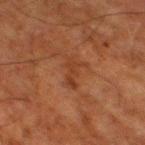Case summary:
• notes: total-body-photography surveillance lesion; no biopsy
• image: total-body-photography crop, ~15 mm field of view
• site: the right thigh
• lighting: cross-polarized
• subject: male, roughly 80 years of age
• lesion diameter: ~3.5 mm (longest diameter)